On the chest · a lesion tile, about 15 mm wide, cut from a 3D total-body photograph · captured under white-light illumination · about 7.5 mm across · a male patient, about 50 years old: 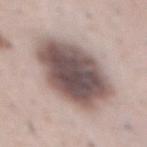Q: What did pathology find?
A: a dysplastic (Clark) nevus